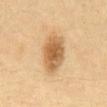A close-up tile cropped from a whole-body skin photograph, about 15 mm across. The patient is a female in their 40s. Located on the abdomen. Approximately 5.5 mm at its widest. Imaged with cross-polarized lighting.